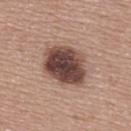Case summary:
* follow-up — imaged on a skin check; not biopsied
* subject — female, aged 58 to 62
* image — ~15 mm crop, total-body skin-cancer survey
* body site — the upper back